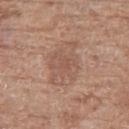• notes · catalogued during a skin exam; not biopsied
• site · the right thigh
• patient · female, roughly 75 years of age
• diameter · ~5 mm (longest diameter)
• illumination · white-light illumination
• automated metrics · a shape-asymmetry score of about 0.25 (0 = symmetric); an average lesion color of about L≈54 a*≈19 b*≈27 (CIELAB), roughly 7 lightness units darker than nearby skin, and a normalized border contrast of about 5; border irregularity of about 3 on a 0–10 scale and a color-variation rating of about 3/10
• image source · total-body-photography crop, ~15 mm field of view